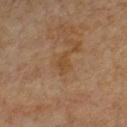The lesion was photographed on a routine skin check and not biopsied; there is no pathology result.
A male subject, roughly 65 years of age.
Located on the chest.
A region of skin cropped from a whole-body photographic capture, roughly 15 mm wide.